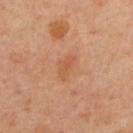Q: Was this lesion biopsied?
A: catalogued during a skin exam; not biopsied
Q: Illumination type?
A: cross-polarized
Q: Lesion size?
A: ~3 mm (longest diameter)
Q: What is the anatomic site?
A: the upper back
Q: Patient demographics?
A: male, approximately 55 years of age
Q: How was this image acquired?
A: 15 mm crop, total-body photography